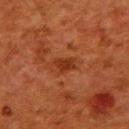– notes · no biopsy performed (imaged during a skin exam)
– body site · the upper back
– automated lesion analysis · an area of roughly 4 mm² and an eccentricity of roughly 0.7; a lesion color around L≈29 a*≈26 b*≈32 in CIELAB, a lesion–skin lightness drop of about 8, and a lesion-to-skin contrast of about 7.5 (normalized; higher = more distinct); a border-irregularity rating of about 3/10 and a within-lesion color-variation index near 2/10; lesion-presence confidence of about 100/100
– illumination · cross-polarized illumination
– subject · female, aged approximately 50
– acquisition · ~15 mm crop, total-body skin-cancer survey